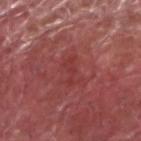Captured during whole-body skin photography for melanoma surveillance; the lesion was not biopsied. The subject is a male aged around 40. This is a white-light tile. A 15 mm close-up tile from a total-body photography series done for melanoma screening. The lesion is on the left forearm.